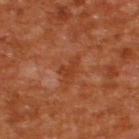Impression: No biopsy was performed on this lesion — it was imaged during a full skin examination and was not determined to be concerning. Background: The subject is a male in their mid-60s. Cropped from a total-body skin-imaging series; the visible field is about 15 mm. Located on the upper back. The recorded lesion diameter is about 3 mm. The tile uses cross-polarized illumination.